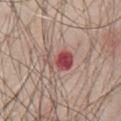Impression: This lesion was catalogued during total-body skin photography and was not selected for biopsy. Background: Captured under white-light illumination. A 15 mm close-up tile from a total-body photography series done for melanoma screening. Longest diameter approximately 3.5 mm. On the chest. A male subject, aged 78 to 82.